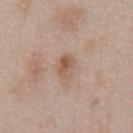{"biopsy_status": "not biopsied; imaged during a skin examination"}